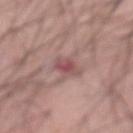Q: Is there a histopathology result?
A: imaged on a skin check; not biopsied
Q: How was this image acquired?
A: total-body-photography crop, ~15 mm field of view
Q: Illumination type?
A: white-light illumination
Q: Patient demographics?
A: male, approximately 60 years of age
Q: Lesion location?
A: the abdomen
Q: Lesion size?
A: ≈2.5 mm
Q: What did automated image analysis measure?
A: a border-irregularity index near 2.5/10, a within-lesion color-variation index near 3/10, and peripheral color asymmetry of about 1